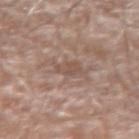biopsy_status: not biopsied; imaged during a skin examination
automated_metrics:
  border_irregularity_0_10: 5.0
  color_variation_0_10: 0.5
  peripheral_color_asymmetry: 0.0
image:
  source: total-body photography crop
  field_of_view_mm: 15
lesion_size:
  long_diameter_mm_approx: 2.5
lighting: white-light
patient:
  sex: male
  age_approx: 70
site: left forearm